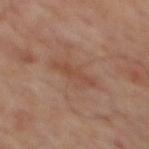Recorded during total-body skin imaging; not selected for excision or biopsy. The patient is a male aged around 65. A roughly 15 mm field-of-view crop from a total-body skin photograph. The lesion-visualizer software estimated a lesion color around L≈45 a*≈20 b*≈28 in CIELAB and a normalized border contrast of about 5.5. The analysis additionally found an automated nevus-likeness rating near 0 out of 100 and a detector confidence of about 100 out of 100 that the crop contains a lesion. Imaged with cross-polarized lighting. The lesion's longest dimension is about 5 mm. The lesion is on the mid back.